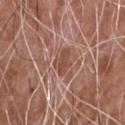<lesion>
  <biopsy_status>not biopsied; imaged during a skin examination</biopsy_status>
  <patient>
    <sex>male</sex>
    <age_approx>75</age_approx>
  </patient>
  <site>chest</site>
  <lighting>white-light</lighting>
  <lesion_size>
    <long_diameter_mm_approx>3.0</long_diameter_mm_approx>
  </lesion_size>
  <image>
    <source>total-body photography crop</source>
    <field_of_view_mm>15</field_of_view_mm>
  </image>
</lesion>